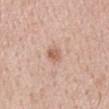Q: Is there a histopathology result?
A: total-body-photography surveillance lesion; no biopsy
Q: Where on the body is the lesion?
A: the mid back
Q: Who is the patient?
A: male, roughly 50 years of age
Q: Illumination type?
A: white-light
Q: How was this image acquired?
A: total-body-photography crop, ~15 mm field of view
Q: Lesion size?
A: ≈2.5 mm